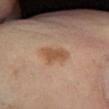Clinical impression: Part of a total-body skin-imaging series; this lesion was reviewed on a skin check and was not flagged for biopsy. Clinical summary: The total-body-photography lesion software estimated an area of roughly 6.5 mm², a shape eccentricity near 0.65, and two-axis asymmetry of about 0.3. The software also gave an average lesion color of about L≈53 a*≈20 b*≈33 (CIELAB), a lesion–skin lightness drop of about 9, and a lesion-to-skin contrast of about 7.5 (normalized; higher = more distinct). The analysis additionally found a border-irregularity rating of about 3/10, internal color variation of about 1.5 on a 0–10 scale, and radial color variation of about 0.5. Approximately 3 mm at its widest. The lesion is on the right lower leg. A 15 mm crop from a total-body photograph taken for skin-cancer surveillance. Imaged with cross-polarized lighting. A female patient in their mid- to late 50s.